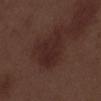Part of a total-body skin-imaging series; this lesion was reviewed on a skin check and was not flagged for biopsy. Located on the left lower leg. Captured under white-light illumination. A 15 mm close-up extracted from a 3D total-body photography capture. The subject is a male approximately 70 years of age. The recorded lesion diameter is about 5 mm. An algorithmic analysis of the crop reported a lesion area of about 13 mm², an eccentricity of roughly 0.75, and a shape-asymmetry score of about 0.4 (0 = symmetric). The analysis additionally found an average lesion color of about L≈24 a*≈19 b*≈19 (CIELAB), about 6 CIELAB-L* units darker than the surrounding skin, and a normalized border contrast of about 7. The analysis additionally found an automated nevus-likeness rating near 5 out of 100 and lesion-presence confidence of about 100/100.